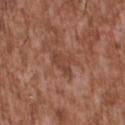Imaged during a routine full-body skin examination; the lesion was not biopsied and no histopathology is available.
From the upper back.
The tile uses white-light illumination.
The lesion's longest dimension is about 3.5 mm.
The patient is a male aged 43 to 47.
This image is a 15 mm lesion crop taken from a total-body photograph.